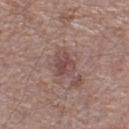Case summary:
* notes: imaged on a skin check; not biopsied
* lighting: white-light illumination
* body site: the left lower leg
* acquisition: ~15 mm crop, total-body skin-cancer survey
* size: ≈3 mm
* patient: male, aged around 70